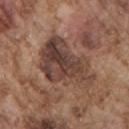Background:
The lesion is on the front of the torso. The lesion's longest dimension is about 7.5 mm. The subject is a male aged 73 to 77. Captured under white-light illumination. Cropped from a total-body skin-imaging series; the visible field is about 15 mm.